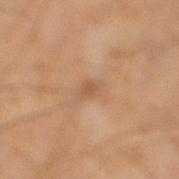Findings:
- follow-up · total-body-photography surveillance lesion; no biopsy
- lighting · cross-polarized
- acquisition · ~15 mm tile from a whole-body skin photo
- anatomic site · the leg
- lesion size · ~2.5 mm (longest diameter)
- patient · male, aged 58 to 62